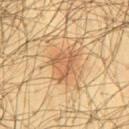biopsy_status: not biopsied; imaged during a skin examination
automated_metrics:
  area_mm2_approx: 8.5
  eccentricity: 0.6
  shape_asymmetry: 0.4
  border_irregularity_0_10: 4.5
  color_variation_0_10: 3.0
  peripheral_color_asymmetry: 1.0
lighting: cross-polarized
lesion_size:
  long_diameter_mm_approx: 4.0
site: lower back
patient:
  sex: male
  age_approx: 45
image:
  source: total-body photography crop
  field_of_view_mm: 15